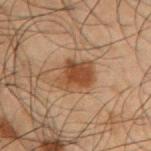Recorded during total-body skin imaging; not selected for excision or biopsy. The recorded lesion diameter is about 4 mm. A male patient, roughly 50 years of age. The total-body-photography lesion software estimated a footprint of about 10 mm² and an eccentricity of roughly 0.55. The software also gave a within-lesion color-variation index near 3.5/10 and radial color variation of about 1. And it measured lesion-presence confidence of about 100/100. A 15 mm close-up tile from a total-body photography series done for melanoma screening. The lesion is located on the arm. Captured under cross-polarized illumination.